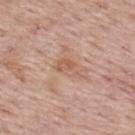Recorded during total-body skin imaging; not selected for excision or biopsy.
The lesion is located on the upper back.
A roughly 15 mm field-of-view crop from a total-body skin photograph.
A male subject about 75 years old.